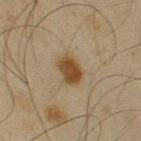Image and clinical context:
A 15 mm close-up extracted from a 3D total-body photography capture. This is a cross-polarized tile. About 3.5 mm across. A male patient, roughly 65 years of age. Located on the right upper arm.